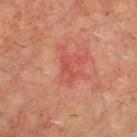Clinical impression: Part of a total-body skin-imaging series; this lesion was reviewed on a skin check and was not flagged for biopsy. Background: Cropped from a total-body skin-imaging series; the visible field is about 15 mm. Imaged with cross-polarized lighting. A male patient in their mid-60s. The lesion is on the back. Measured at roughly 3 mm in maximum diameter.